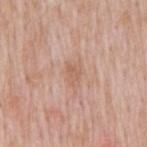workup: imaged on a skin check; not biopsied | TBP lesion metrics: a lesion area of about 4.5 mm², an outline eccentricity of about 0.75 (0 = round, 1 = elongated), and two-axis asymmetry of about 0.3 | anatomic site: the mid back | acquisition: ~15 mm crop, total-body skin-cancer survey | patient: male, approximately 50 years of age.